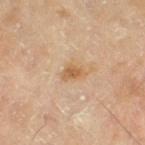notes: total-body-photography surveillance lesion; no biopsy
image source: ~15 mm tile from a whole-body skin photo
anatomic site: the right thigh
patient: male, in their mid- to late 60s
illumination: cross-polarized illumination
diameter: about 2.5 mm